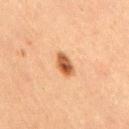workup — imaged on a skin check; not biopsied | image-analysis metrics — a lesion area of about 5 mm², an eccentricity of roughly 0.85, and two-axis asymmetry of about 0.25; roughly 14 lightness units darker than nearby skin and a normalized lesion–skin contrast near 10; a border-irregularity rating of about 2.5/10, a color-variation rating of about 5.5/10, and radial color variation of about 2 | site — the lower back | size — ≈3.5 mm | acquisition — ~15 mm crop, total-body skin-cancer survey | patient — female, aged 38 to 42 | lighting — cross-polarized.